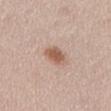Findings:
* notes — imaged on a skin check; not biopsied
* diameter — about 3 mm
* illumination — white-light
* image — 15 mm crop, total-body photography
* subject — female, about 65 years old
* site — the abdomen
* image-analysis metrics — an average lesion color of about L≈57 a*≈19 b*≈29 (CIELAB) and about 12 CIELAB-L* units darker than the surrounding skin; border irregularity of about 2 on a 0–10 scale and a peripheral color-asymmetry measure near 0.5; a classifier nevus-likeness of about 95/100 and a detector confidence of about 100 out of 100 that the crop contains a lesion